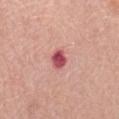{"biopsy_status": "not biopsied; imaged during a skin examination", "image": {"source": "total-body photography crop", "field_of_view_mm": 15}, "lighting": "white-light", "site": "back", "patient": {"sex": "male", "age_approx": 80}, "automated_metrics": {"nevus_likeness_0_100": 0, "lesion_detection_confidence_0_100": 100}}